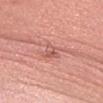Context: On the head or neck. A male patient, aged around 30. Measured at roughly 2.5 mm in maximum diameter. An algorithmic analysis of the crop reported a border-irregularity index near 4.5/10 and a within-lesion color-variation index near 3.5/10. The analysis additionally found a classifier nevus-likeness of about 0/100 and a lesion-detection confidence of about 95/100. A 15 mm close-up tile from a total-body photography series done for melanoma screening.